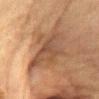Imaged during a routine full-body skin examination; the lesion was not biopsied and no histopathology is available. Imaged with cross-polarized lighting. The subject is a female in their 40s. Cropped from a total-body skin-imaging series; the visible field is about 15 mm. The lesion is located on the abdomen. The lesion's longest dimension is about 6.5 mm.